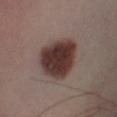Assessment:
Recorded during total-body skin imaging; not selected for excision or biopsy.
Context:
On the left lower leg. A male patient, aged approximately 40. A 15 mm crop from a total-body photograph taken for skin-cancer surveillance.